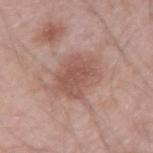biopsy_status: not biopsied; imaged during a skin examination
automated_metrics:
  cielab_L: 53
  cielab_a: 22
  cielab_b: 25
  vs_skin_darker_L: 9.0
  vs_skin_contrast_norm: 6.5
  border_irregularity_0_10: 3.0
  color_variation_0_10: 2.5
  peripheral_color_asymmetry: 1.0
  nevus_likeness_0_100: 85
patient:
  sex: male
  age_approx: 45
site: left upper arm
lighting: white-light
image:
  source: total-body photography crop
  field_of_view_mm: 15